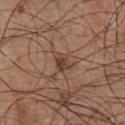acquisition — 15 mm crop, total-body photography
site — the chest
subject — male, aged 53–57
automated metrics — a lesion color around L≈42 a*≈18 b*≈26 in CIELAB, roughly 9 lightness units darker than nearby skin, and a lesion-to-skin contrast of about 7.5 (normalized; higher = more distinct); a border-irregularity index near 3.5/10, a within-lesion color-variation index near 3.5/10, and a peripheral color-asymmetry measure near 1